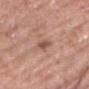Findings:
– diameter: ≈2.5 mm
– location: the chest
– illumination: white-light
– image: ~15 mm crop, total-body skin-cancer survey
– patient: male, aged 58 to 62
– automated lesion analysis: a footprint of about 3.5 mm², an outline eccentricity of about 0.8 (0 = round, 1 = elongated), and a shape-asymmetry score of about 0.3 (0 = symmetric); an average lesion color of about L≈53 a*≈21 b*≈26 (CIELAB) and a lesion–skin lightness drop of about 10; a border-irregularity rating of about 2.5/10, a within-lesion color-variation index near 2.5/10, and a peripheral color-asymmetry measure near 0.5